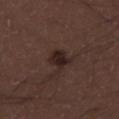{
  "biopsy_status": "not biopsied; imaged during a skin examination",
  "image": {
    "source": "total-body photography crop",
    "field_of_view_mm": 15
  },
  "lighting": "white-light",
  "lesion_size": {
    "long_diameter_mm_approx": 3.0
  },
  "site": "right lower leg",
  "patient": {
    "sex": "male",
    "age_approx": 30
  }
}